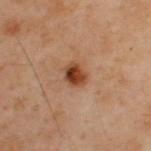Imaged during a routine full-body skin examination; the lesion was not biopsied and no histopathology is available. From the upper back. The subject is a male roughly 50 years of age. A roughly 15 mm field-of-view crop from a total-body skin photograph.